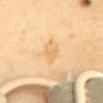Imaged during a routine full-body skin examination; the lesion was not biopsied and no histopathology is available.
This is a cross-polarized tile.
Approximately 3 mm at its widest.
A 15 mm close-up extracted from a 3D total-body photography capture.
The lesion-visualizer software estimated an area of roughly 4.5 mm² and an eccentricity of roughly 0.8. The analysis additionally found an automated nevus-likeness rating near 0 out of 100 and a detector confidence of about 100 out of 100 that the crop contains a lesion.
A female subject approximately 55 years of age.
From the mid back.